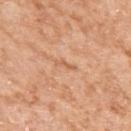Impression: The lesion was tiled from a total-body skin photograph and was not biopsied. Image and clinical context: The subject is a female aged around 75. A 15 mm close-up extracted from a 3D total-body photography capture. The lesion is on the left upper arm.